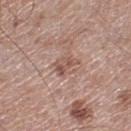No biopsy was performed on this lesion — it was imaged during a full skin examination and was not determined to be concerning. Automated image analysis of the tile measured an outline eccentricity of about 0.8 (0 = round, 1 = elongated) and a shape-asymmetry score of about 0.45 (0 = symmetric). The analysis additionally found a mean CIELAB color near L≈53 a*≈20 b*≈25, about 9 CIELAB-L* units darker than the surrounding skin, and a lesion-to-skin contrast of about 6.5 (normalized; higher = more distinct). It also reported a border-irregularity rating of about 4.5/10, a within-lesion color-variation index near 3.5/10, and peripheral color asymmetry of about 1.5. This is a white-light tile. Located on the right lower leg. This image is a 15 mm lesion crop taken from a total-body photograph. A male subject aged 48–52. Measured at roughly 3 mm in maximum diameter.